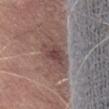workup = imaged on a skin check; not biopsied.